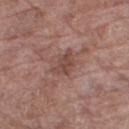The recorded lesion diameter is about 3 mm.
A female subject, about 70 years old.
The tile uses white-light illumination.
A close-up tile cropped from a whole-body skin photograph, about 15 mm across.
The lesion is on the leg.